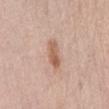The lesion was tiled from a total-body skin photograph and was not biopsied.
A male patient aged around 60.
The tile uses white-light illumination.
This image is a 15 mm lesion crop taken from a total-body photograph.
On the abdomen.